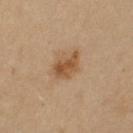notes: catalogued during a skin exam; not biopsied
acquisition: ~15 mm tile from a whole-body skin photo
diameter: ≈4 mm
body site: the arm
subject: female, roughly 55 years of age
tile lighting: cross-polarized illumination